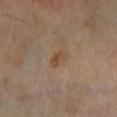workup = no biopsy performed (imaged during a skin exam)
patient = male, aged around 70
body site = the leg
acquisition = ~15 mm tile from a whole-body skin photo
lesion diameter = ~2.5 mm (longest diameter)
tile lighting = cross-polarized